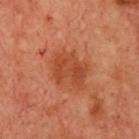  biopsy_status: not biopsied; imaged during a skin examination
  patient:
    sex: female
    age_approx: 55
  site: chest
  image:
    source: total-body photography crop
    field_of_view_mm: 15